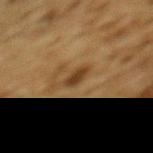<tbp_lesion>
<biopsy_status>not biopsied; imaged during a skin examination</biopsy_status>
<image>
  <source>total-body photography crop</source>
  <field_of_view_mm>15</field_of_view_mm>
</image>
<site>upper back</site>
<patient>
  <sex>male</sex>
  <age_approx>85+</age_approx>
</patient>
</tbp_lesion>